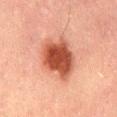Clinical impression:
Part of a total-body skin-imaging series; this lesion was reviewed on a skin check and was not flagged for biopsy.
Acquisition and patient details:
The lesion is on the lower back. The lesion's longest dimension is about 6 mm. The total-body-photography lesion software estimated a nevus-likeness score of about 100/100 and a lesion-detection confidence of about 100/100. Captured under cross-polarized illumination. A region of skin cropped from a whole-body photographic capture, roughly 15 mm wide. A male patient, aged around 50.